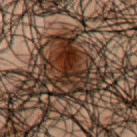Background:
This is a cross-polarized tile. A male subject, roughly 50 years of age. Cropped from a total-body skin-imaging series; the visible field is about 15 mm. The lesion is on the chest. About 6.5 mm across.